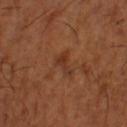A close-up tile cropped from a whole-body skin photograph, about 15 mm across. Located on the left lower leg. Captured under cross-polarized illumination. A male patient, aged approximately 65.